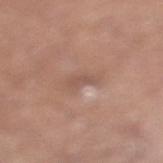The tile uses white-light illumination. The lesion is located on the right lower leg. A roughly 15 mm field-of-view crop from a total-body skin photograph. A male subject aged 43 to 47. About 3 mm across.